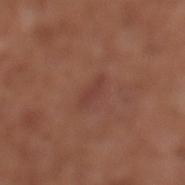Recorded during total-body skin imaging; not selected for excision or biopsy. The patient is a male roughly 75 years of age. From the leg. The total-body-photography lesion software estimated a footprint of about 3 mm², an outline eccentricity of about 0.95 (0 = round, 1 = elongated), and a symmetry-axis asymmetry near 0.35. The software also gave a border-irregularity rating of about 3.5/10 and a color-variation rating of about 0/10. This is a white-light tile. About 3 mm across. A close-up tile cropped from a whole-body skin photograph, about 15 mm across.